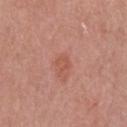  biopsy_status: not biopsied; imaged during a skin examination
  image:
    source: total-body photography crop
    field_of_view_mm: 15
  patient:
    sex: female
    age_approx: 40
  lesion_size:
    long_diameter_mm_approx: 2.0
  automated_metrics:
    area_mm2_approx: 2.5
    shape_asymmetry: 0.3
    cielab_L: 54
    cielab_a: 27
    cielab_b: 30
    vs_skin_darker_L: 6.0
    vs_skin_contrast_norm: 5.0
    border_irregularity_0_10: 3.0
    color_variation_0_10: 0.5
    peripheral_color_asymmetry: 0.0
  site: head or neck
  lighting: white-light